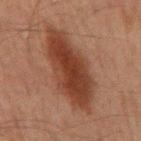Case summary:
- follow-up — catalogued during a skin exam; not biopsied
- illumination — cross-polarized
- image-analysis metrics — an area of roughly 28 mm² and an outline eccentricity of about 0.95 (0 = round, 1 = elongated); a lesion color around L≈30 a*≈19 b*≈24 in CIELAB, about 10 CIELAB-L* units darker than the surrounding skin, and a normalized border contrast of about 10
- patient — male, in their 60s
- location — the mid back
- diameter — about 10.5 mm
- acquisition — ~15 mm crop, total-body skin-cancer survey